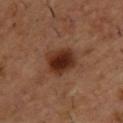Clinical impression: Part of a total-body skin-imaging series; this lesion was reviewed on a skin check and was not flagged for biopsy. Background: Captured under cross-polarized illumination. Automated image analysis of the tile measured an area of roughly 11 mm² and two-axis asymmetry of about 0.2. The software also gave an average lesion color of about L≈30 a*≈21 b*≈27 (CIELAB), roughly 12 lightness units darker than nearby skin, and a normalized border contrast of about 11.5. A male subject, aged around 55. The lesion is on the upper back. The lesion's longest dimension is about 4 mm. Cropped from a total-body skin-imaging series; the visible field is about 15 mm.